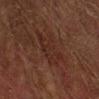Clinical impression: Recorded during total-body skin imaging; not selected for excision or biopsy. Background: The tile uses cross-polarized illumination. A male patient, approximately 75 years of age. From the left forearm. A region of skin cropped from a whole-body photographic capture, roughly 15 mm wide.